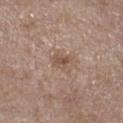No biopsy was performed on this lesion — it was imaged during a full skin examination and was not determined to be concerning.